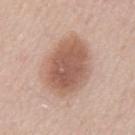biopsy_status: not biopsied; imaged during a skin examination
site: mid back
lighting: white-light
image:
  source: total-body photography crop
  field_of_view_mm: 15
patient:
  sex: male
  age_approx: 65